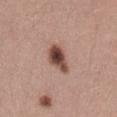The lesion was tiled from a total-body skin photograph and was not biopsied.
Captured under white-light illumination.
On the lower back.
About 4 mm across.
Cropped from a whole-body photographic skin survey; the tile spans about 15 mm.
A female subject, approximately 30 years of age.